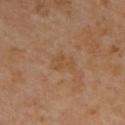Clinical impression:
The lesion was tiled from a total-body skin photograph and was not biopsied.
Clinical summary:
Located on the back. About 2.5 mm across. A male subject about 50 years old. Cropped from a whole-body photographic skin survey; the tile spans about 15 mm.